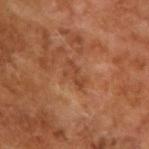The lesion was photographed on a routine skin check and not biopsied; there is no pathology result.